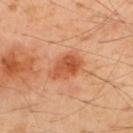{
  "biopsy_status": "not biopsied; imaged during a skin examination",
  "lighting": "cross-polarized",
  "site": "upper back",
  "patient": {
    "sex": "male",
    "age_approx": 50
  },
  "image": {
    "source": "total-body photography crop",
    "field_of_view_mm": 15
  },
  "automated_metrics": {
    "cielab_L": 55,
    "cielab_a": 30,
    "cielab_b": 39,
    "vs_skin_darker_L": 11.0,
    "vs_skin_contrast_norm": 8.0,
    "lesion_detection_confidence_0_100": 100
  }
}